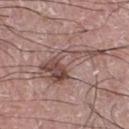No biopsy was performed on this lesion — it was imaged during a full skin examination and was not determined to be concerning. The lesion-visualizer software estimated a footprint of about 15 mm², an eccentricity of roughly 0.9, and a shape-asymmetry score of about 0.6 (0 = symmetric). The analysis additionally found an average lesion color of about L≈48 a*≈18 b*≈22 (CIELAB), a lesion–skin lightness drop of about 11, and a normalized border contrast of about 7.5. The software also gave a color-variation rating of about 9/10 and peripheral color asymmetry of about 4. Cropped from a total-body skin-imaging series; the visible field is about 15 mm. The patient is a male in their mid- to late 70s. The lesion is located on the left thigh. The tile uses white-light illumination. About 8 mm across.